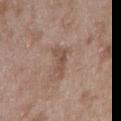Recorded during total-body skin imaging; not selected for excision or biopsy. The recorded lesion diameter is about 3.5 mm. A close-up tile cropped from a whole-body skin photograph, about 15 mm across. On the right upper arm. This is a white-light tile. The subject is a male about 50 years old. Automated tile analysis of the lesion measured an area of roughly 5 mm², a shape eccentricity near 0.85, and two-axis asymmetry of about 0.5. It also reported an average lesion color of about L≈50 a*≈17 b*≈26 (CIELAB), roughly 8 lightness units darker than nearby skin, and a normalized lesion–skin contrast near 6.